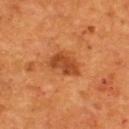Imaged during a routine full-body skin examination; the lesion was not biopsied and no histopathology is available. A male subject, about 55 years old. Imaged with cross-polarized lighting. The lesion is located on the upper back. Automated image analysis of the tile measured a shape eccentricity near 0.8. And it measured a lesion color around L≈43 a*≈28 b*≈39 in CIELAB, roughly 10 lightness units darker than nearby skin, and a normalized border contrast of about 8. It also reported border irregularity of about 3.5 on a 0–10 scale, a color-variation rating of about 2.5/10, and radial color variation of about 1. It also reported an automated nevus-likeness rating near 25 out of 100 and a detector confidence of about 100 out of 100 that the crop contains a lesion. About 4 mm across. A 15 mm close-up tile from a total-body photography series done for melanoma screening.